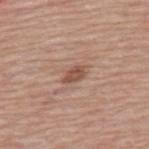Notes:
• subject: male, approximately 80 years of age
• acquisition: total-body-photography crop, ~15 mm field of view
• anatomic site: the upper back
• tile lighting: white-light illumination
• lesion size: ≈2.5 mm